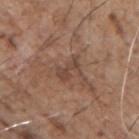biopsy status: total-body-photography surveillance lesion; no biopsy | TBP lesion metrics: a footprint of about 5.5 mm², an eccentricity of roughly 0.8, and a shape-asymmetry score of about 0.45 (0 = symmetric) | subject: male, aged around 70 | lighting: white-light illumination | lesion size: ~3.5 mm (longest diameter) | location: the right upper arm | image source: 15 mm crop, total-body photography.